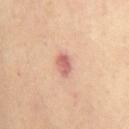{"biopsy_status": "not biopsied; imaged during a skin examination", "patient": {"sex": "female", "age_approx": 45}, "lesion_size": {"long_diameter_mm_approx": 2.5}, "automated_metrics": {"border_irregularity_0_10": 1.5, "color_variation_0_10": 2.5, "peripheral_color_asymmetry": 1.0, "nevus_likeness_0_100": 5, "lesion_detection_confidence_0_100": 100}, "site": "chest", "image": {"source": "total-body photography crop", "field_of_view_mm": 15}, "lighting": "cross-polarized"}